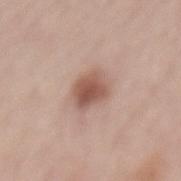biopsy status: total-body-photography surveillance lesion; no biopsy | lesion diameter: ≈3.5 mm | patient: female, aged 63–67 | image source: total-body-photography crop, ~15 mm field of view | body site: the back | automated metrics: a border-irregularity rating of about 2/10 and a color-variation rating of about 4.5/10; a nevus-likeness score of about 90/100 and a detector confidence of about 100 out of 100 that the crop contains a lesion | tile lighting: white-light.